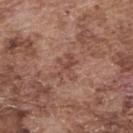Captured during whole-body skin photography for melanoma surveillance; the lesion was not biopsied. A male patient roughly 75 years of age. A lesion tile, about 15 mm wide, cut from a 3D total-body photograph. Captured under white-light illumination. Located on the upper back. The lesion's longest dimension is about 3.5 mm.